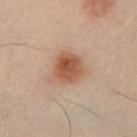Clinical impression: The lesion was photographed on a routine skin check and not biopsied; there is no pathology result. Background: Longest diameter approximately 3.5 mm. This is a cross-polarized tile. An algorithmic analysis of the crop reported an area of roughly 10 mm², an eccentricity of roughly 0.4, and a shape-asymmetry score of about 0.15 (0 = symmetric). It also reported an average lesion color of about L≈43 a*≈18 b*≈26 (CIELAB) and a lesion–skin lightness drop of about 10. The analysis additionally found a border-irregularity index near 1.5/10, a within-lesion color-variation index near 4/10, and peripheral color asymmetry of about 1. On the left lower leg. A 15 mm close-up extracted from a 3D total-body photography capture. The patient is a male approximately 50 years of age.